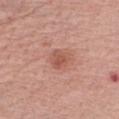Context:
Imaged with white-light lighting. On the arm. A close-up tile cropped from a whole-body skin photograph, about 15 mm across. The subject is a female about 65 years old. About 3 mm across. Automated image analysis of the tile measured an average lesion color of about L≈55 a*≈25 b*≈29 (CIELAB) and a normalized border contrast of about 6.5. And it measured a border-irregularity rating of about 2/10, a color-variation rating of about 3/10, and peripheral color asymmetry of about 1. And it measured an automated nevus-likeness rating near 30 out of 100 and a detector confidence of about 100 out of 100 that the crop contains a lesion.